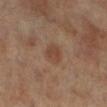workup = catalogued during a skin exam; not biopsied
anatomic site = the left leg
image-analysis metrics = a mean CIELAB color near L≈43 a*≈18 b*≈27 and about 7 CIELAB-L* units darker than the surrounding skin
size = about 3 mm
patient = female, in their mid-60s
lighting = cross-polarized
image = ~15 mm tile from a whole-body skin photo